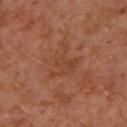Part of a total-body skin-imaging series; this lesion was reviewed on a skin check and was not flagged for biopsy.
Captured under cross-polarized illumination.
Located on the left arm.
An algorithmic analysis of the crop reported a footprint of about 10 mm² and a shape eccentricity near 0.45. The software also gave a border-irregularity index near 6.5/10 and peripheral color asymmetry of about 1. And it measured a classifier nevus-likeness of about 0/100 and lesion-presence confidence of about 100/100.
The subject is a male in their 30s.
A region of skin cropped from a whole-body photographic capture, roughly 15 mm wide.